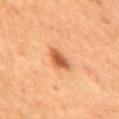No biopsy was performed on this lesion — it was imaged during a full skin examination and was not determined to be concerning.
The tile uses cross-polarized illumination.
The lesion is on the right upper arm.
A close-up tile cropped from a whole-body skin photograph, about 15 mm across.
A female patient aged 58–62.
Automated image analysis of the tile measured a mean CIELAB color near L≈47 a*≈23 b*≈35 and a lesion-to-skin contrast of about 9 (normalized; higher = more distinct).